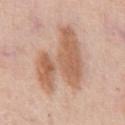Q: Was a biopsy performed?
A: imaged on a skin check; not biopsied
Q: How was this image acquired?
A: ~15 mm crop, total-body skin-cancer survey
Q: What is the anatomic site?
A: the front of the torso
Q: Who is the patient?
A: male, about 50 years old